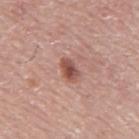The lesion was photographed on a routine skin check and not biopsied; there is no pathology result. An algorithmic analysis of the crop reported roughly 13 lightness units darker than nearby skin and a normalized border contrast of about 9. The analysis additionally found a border-irregularity index near 2.5/10 and peripheral color asymmetry of about 1. The analysis additionally found a detector confidence of about 100 out of 100 that the crop contains a lesion. From the mid back. Cropped from a whole-body photographic skin survey; the tile spans about 15 mm. The subject is a male in their mid-70s.